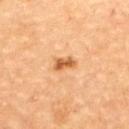{
  "image": {
    "source": "total-body photography crop",
    "field_of_view_mm": 15
  },
  "lesion_size": {
    "long_diameter_mm_approx": 3.0
  },
  "site": "upper back",
  "patient": {
    "sex": "male",
    "age_approx": "85+"
  }
}